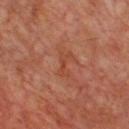Case summary:
- biopsy status · imaged on a skin check; not biopsied
- location · the front of the torso
- subject · male, aged approximately 65
- image source · ~15 mm tile from a whole-body skin photo